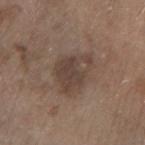Recorded during total-body skin imaging; not selected for excision or biopsy.
The lesion is located on the right forearm.
A male subject about 65 years old.
Automated image analysis of the tile measured a footprint of about 15 mm², a shape eccentricity near 0.6, and a shape-asymmetry score of about 0.3 (0 = symmetric). And it measured a mean CIELAB color near L≈42 a*≈14 b*≈23, a lesion–skin lightness drop of about 8, and a normalized border contrast of about 6.5. The software also gave a border-irregularity index near 3/10 and radial color variation of about 1.5. The analysis additionally found a classifier nevus-likeness of about 5/100 and a detector confidence of about 100 out of 100 that the crop contains a lesion.
A 15 mm close-up tile from a total-body photography series done for melanoma screening.
Approximately 5 mm at its widest.
The tile uses white-light illumination.